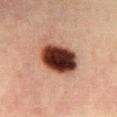Case summary:
• imaging modality — total-body-photography crop, ~15 mm field of view
• location — the abdomen
• illumination — cross-polarized illumination
• patient — female, aged around 40
• automated lesion analysis — a footprint of about 17 mm² and an eccentricity of roughly 0.7; a border-irregularity index near 1.5/10 and a peripheral color-asymmetry measure near 2.5; a classifier nevus-likeness of about 95/100 and a detector confidence of about 100 out of 100 that the crop contains a lesion
• diameter — ≈5.5 mm